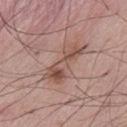Findings:
• follow-up: no biopsy performed (imaged during a skin exam)
• subject: male, aged 53 to 57
• body site: the abdomen
• acquisition: 15 mm crop, total-body photography